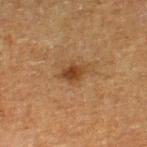Findings:
• follow-up · catalogued during a skin exam; not biopsied
• patient · male, roughly 75 years of age
• image · 15 mm crop, total-body photography
• illumination · cross-polarized
• diameter · ≈3 mm
• location · the right lower leg